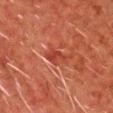{"biopsy_status": "not biopsied; imaged during a skin examination", "lesion_size": {"long_diameter_mm_approx": 2.5}, "lighting": "cross-polarized", "image": {"source": "total-body photography crop", "field_of_view_mm": 15}, "patient": {"sex": "male", "age_approx": 60}, "site": "chest"}